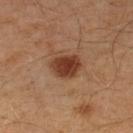{"biopsy_status": "not biopsied; imaged during a skin examination", "patient": {"sex": "male", "age_approx": 55}, "site": "left lower leg", "image": {"source": "total-body photography crop", "field_of_view_mm": 15}, "automated_metrics": {"area_mm2_approx": 8.0, "eccentricity": 0.6, "shape_asymmetry": 0.2, "vs_skin_darker_L": 13.0, "vs_skin_contrast_norm": 11.0}, "lesion_size": {"long_diameter_mm_approx": 3.5}, "lighting": "cross-polarized"}